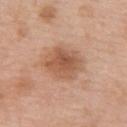This lesion was catalogued during total-body skin photography and was not selected for biopsy. The lesion's longest dimension is about 5 mm. A 15 mm close-up extracted from a 3D total-body photography capture. From the chest. The tile uses white-light illumination. A female subject roughly 50 years of age. The lesion-visualizer software estimated a footprint of about 15 mm², a shape eccentricity near 0.6, and a shape-asymmetry score of about 0.15 (0 = symmetric). The software also gave an average lesion color of about L≈56 a*≈22 b*≈32 (CIELAB), about 10 CIELAB-L* units darker than the surrounding skin, and a normalized border contrast of about 7.5. It also reported a border-irregularity rating of about 1.5/10, internal color variation of about 4.5 on a 0–10 scale, and a peripheral color-asymmetry measure near 1.5. It also reported a classifier nevus-likeness of about 85/100 and a lesion-detection confidence of about 100/100.